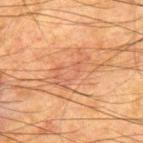body site: the upper back | tile lighting: cross-polarized illumination | image-analysis metrics: a border-irregularity index near 7/10 and radial color variation of about 1 | image source: ~15 mm crop, total-body skin-cancer survey | patient: male, roughly 65 years of age.